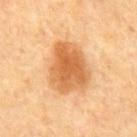  lighting: cross-polarized
  site: abdomen
  lesion_size:
    long_diameter_mm_approx: 6.0
  patient:
    sex: male
    age_approx: 65
  automated_metrics:
    area_mm2_approx: 20.0
    eccentricity: 0.6
    shape_asymmetry: 0.25
    color_variation_0_10: 4.0
    peripheral_color_asymmetry: 1.5
    nevus_likeness_0_100: 95
  image:
    source: total-body photography crop
    field_of_view_mm: 15